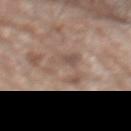Clinical impression: Imaged during a routine full-body skin examination; the lesion was not biopsied and no histopathology is available. Background: The tile uses white-light illumination. A roughly 15 mm field-of-view crop from a total-body skin photograph. The recorded lesion diameter is about 6.5 mm. The lesion is on the mid back. The subject is a male roughly 80 years of age.